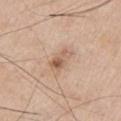Imaged during a routine full-body skin examination; the lesion was not biopsied and no histopathology is available.
The subject is a male aged around 70.
Imaged with white-light lighting.
A roughly 15 mm field-of-view crop from a total-body skin photograph.
The recorded lesion diameter is about 3 mm.
Located on the chest.